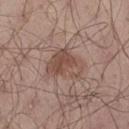Assessment:
The lesion was tiled from a total-body skin photograph and was not biopsied.
Image and clinical context:
A lesion tile, about 15 mm wide, cut from a 3D total-body photograph. The lesion is on the leg. The patient is a male about 55 years old. The lesion-visualizer software estimated a nevus-likeness score of about 50/100 and lesion-presence confidence of about 100/100. This is a white-light tile.